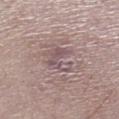  biopsy_status: not biopsied; imaged during a skin examination
  site: left lower leg
  image:
    source: total-body photography crop
    field_of_view_mm: 15
  lighting: white-light
  lesion_size:
    long_diameter_mm_approx: 3.5
  automated_metrics:
    vs_skin_darker_L: 8.0
    vs_skin_contrast_norm: 6.0
    nevus_likeness_0_100: 0
    lesion_detection_confidence_0_100: 65
  patient:
    sex: male
    age_approx: 65